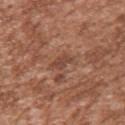The lesion was tiled from a total-body skin photograph and was not biopsied. On the left upper arm. The total-body-photography lesion software estimated about 8 CIELAB-L* units darker than the surrounding skin and a normalized lesion–skin contrast near 6.5. And it measured a border-irregularity rating of about 3.5/10, internal color variation of about 1.5 on a 0–10 scale, and radial color variation of about 0.5. The analysis additionally found a classifier nevus-likeness of about 0/100. Cropped from a whole-body photographic skin survey; the tile spans about 15 mm. This is a white-light tile. A male patient, aged around 45. About 3 mm across.